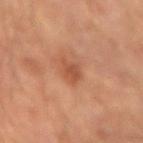Case summary:
– notes — total-body-photography surveillance lesion; no biopsy
– patient — male, in their mid-40s
– lesion size — ≈2.5 mm
– location — the left forearm
– lighting — cross-polarized
– imaging modality — ~15 mm tile from a whole-body skin photo